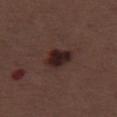Findings:
• workup: total-body-photography surveillance lesion; no biopsy
• image: ~15 mm tile from a whole-body skin photo
• automated metrics: a mean CIELAB color near L≈23 a*≈17 b*≈17 and a lesion-to-skin contrast of about 12 (normalized; higher = more distinct); a border-irregularity rating of about 1.5/10, a within-lesion color-variation index near 4/10, and peripheral color asymmetry of about 1.5
• patient: female, roughly 50 years of age
• body site: the right thigh
• illumination: white-light illumination
• lesion diameter: about 4 mm